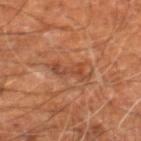Impression:
The lesion was tiled from a total-body skin photograph and was not biopsied.
Clinical summary:
A region of skin cropped from a whole-body photographic capture, roughly 15 mm wide. Located on the leg. The patient is a male roughly 60 years of age.